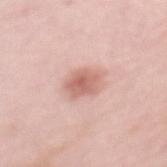<case>
  <biopsy_status>not biopsied; imaged during a skin examination</biopsy_status>
  <patient>
    <sex>female</sex>
    <age_approx>65</age_approx>
  </patient>
  <lighting>white-light</lighting>
  <lesion_size>
    <long_diameter_mm_approx>3.5</long_diameter_mm_approx>
  </lesion_size>
  <automated_metrics>
    <border_irregularity_0_10>2.0</border_irregularity_0_10>
  </automated_metrics>
  <site>mid back</site>
  <image>
    <source>total-body photography crop</source>
    <field_of_view_mm>15</field_of_view_mm>
  </image>
</case>